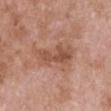– notes — catalogued during a skin exam; not biopsied
– lighting — white-light
– diameter — ~6 mm (longest diameter)
– body site — the chest
– patient — female, aged 73–77
– image source — 15 mm crop, total-body photography